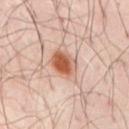Clinical impression:
This lesion was catalogued during total-body skin photography and was not selected for biopsy.
Background:
A male subject roughly 50 years of age. The lesion's longest dimension is about 4 mm. A 15 mm crop from a total-body photograph taken for skin-cancer surveillance. Located on the front of the torso.